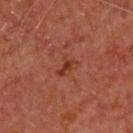Notes:
* follow-up · imaged on a skin check; not biopsied
* lighting · cross-polarized
* acquisition · ~15 mm tile from a whole-body skin photo
* lesion diameter · ≈2.5 mm
* site · the upper back
* patient · male, roughly 65 years of age